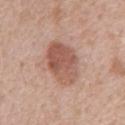Notes:
• follow-up · catalogued during a skin exam; not biopsied
• patient · male, about 60 years old
• lighting · white-light illumination
• anatomic site · the front of the torso
• imaging modality · total-body-photography crop, ~15 mm field of view
• automated metrics · a footprint of about 16 mm², an eccentricity of roughly 0.75, and a symmetry-axis asymmetry near 0.15; a border-irregularity index near 1.5/10 and a within-lesion color-variation index near 5/10